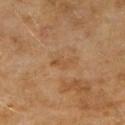Findings:
• notes: no biopsy performed (imaged during a skin exam)
• automated metrics: an outline eccentricity of about 0.9 (0 = round, 1 = elongated); a lesion color around L≈46 a*≈18 b*≈34 in CIELAB, roughly 5 lightness units darker than nearby skin, and a lesion-to-skin contrast of about 5 (normalized; higher = more distinct); a border-irregularity rating of about 4.5/10, internal color variation of about 0 on a 0–10 scale, and peripheral color asymmetry of about 0; a nevus-likeness score of about 0/100 and lesion-presence confidence of about 100/100
• imaging modality: ~15 mm tile from a whole-body skin photo
• illumination: cross-polarized illumination
• subject: female, aged around 60
• location: the right upper arm
• lesion size: ~3 mm (longest diameter)